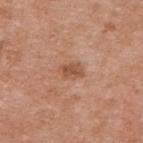Recorded during total-body skin imaging; not selected for excision or biopsy.
An algorithmic analysis of the crop reported an area of roughly 4 mm², an eccentricity of roughly 0.8, and a symmetry-axis asymmetry near 0.2. The analysis additionally found an average lesion color of about L≈53 a*≈23 b*≈33 (CIELAB), about 9 CIELAB-L* units darker than the surrounding skin, and a normalized border contrast of about 7. The software also gave a classifier nevus-likeness of about 35/100.
A male subject, approximately 55 years of age.
Captured under white-light illumination.
A close-up tile cropped from a whole-body skin photograph, about 15 mm across.
The lesion is located on the upper back.